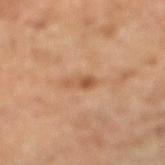Part of a total-body skin-imaging series; this lesion was reviewed on a skin check and was not flagged for biopsy.
Cropped from a total-body skin-imaging series; the visible field is about 15 mm.
About 3 mm across.
The lesion is on the right lower leg.
A female patient, aged around 70.
Imaged with cross-polarized lighting.